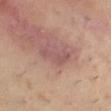No biopsy was performed on this lesion — it was imaged during a full skin examination and was not determined to be concerning.
On the right lower leg.
Captured under cross-polarized illumination.
The lesion's longest dimension is about 4.5 mm.
A region of skin cropped from a whole-body photographic capture, roughly 15 mm wide.
A female subject, aged 43–47.